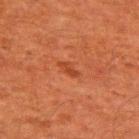  biopsy_status: not biopsied; imaged during a skin examination
  image:
    source: total-body photography crop
    field_of_view_mm: 15
  patient:
    sex: male
    age_approx: 60
  lighting: cross-polarized
  lesion_size:
    long_diameter_mm_approx: 2.5
  automated_metrics:
    area_mm2_approx: 2.5
    eccentricity: 0.9
    shape_asymmetry: 0.3
    cielab_L: 35
    cielab_a: 27
    cielab_b: 33
    vs_skin_darker_L: 7.0
    vs_skin_contrast_norm: 6.0
    border_irregularity_0_10: 3.0
    color_variation_0_10: 0.0
    peripheral_color_asymmetry: 0.0
  site: upper back